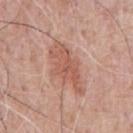workup: total-body-photography surveillance lesion; no biopsy | subject: male, aged 58 to 62 | automated metrics: a border-irregularity rating of about 5.5/10, internal color variation of about 3 on a 0–10 scale, and a peripheral color-asymmetry measure near 1; a classifier nevus-likeness of about 20/100 | acquisition: 15 mm crop, total-body photography | anatomic site: the front of the torso | size: ≈6 mm | lighting: white-light.